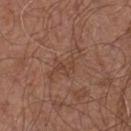subject=male, roughly 55 years of age; automated metrics=a border-irregularity rating of about 9.5/10, internal color variation of about 1 on a 0–10 scale, and a peripheral color-asymmetry measure near 0.5; site=the chest; imaging modality=~15 mm tile from a whole-body skin photo.